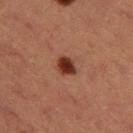The lesion was tiled from a total-body skin photograph and was not biopsied. A female subject roughly 40 years of age. Measured at roughly 2.5 mm in maximum diameter. Automated image analysis of the tile measured a within-lesion color-variation index near 3.5/10 and a peripheral color-asymmetry measure near 1. It also reported a classifier nevus-likeness of about 100/100 and lesion-presence confidence of about 100/100. The tile uses cross-polarized illumination. From the left thigh. A region of skin cropped from a whole-body photographic capture, roughly 15 mm wide.